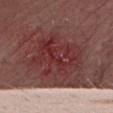The lesion was tiled from a total-body skin photograph and was not biopsied. This image is a 15 mm lesion crop taken from a total-body photograph. The lesion-visualizer software estimated a symmetry-axis asymmetry near 0.3. The software also gave a nevus-likeness score of about 0/100 and lesion-presence confidence of about 75/100. The lesion is on the leg. A female patient aged approximately 30. Measured at roughly 7.5 mm in maximum diameter.